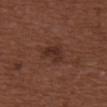Notes:
* notes · no biopsy performed (imaged during a skin exam)
* image-analysis metrics · a lesion area of about 6 mm², an eccentricity of roughly 0.85, and two-axis asymmetry of about 0.35; a lesion–skin lightness drop of about 7 and a normalized border contrast of about 7; a classifier nevus-likeness of about 20/100
* image · ~15 mm tile from a whole-body skin photo
* body site · the back
* illumination · white-light illumination
* patient · male, aged approximately 30
* lesion diameter · about 3.5 mm